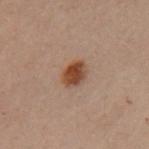Recorded during total-body skin imaging; not selected for excision or biopsy.
Longest diameter approximately 3 mm.
Captured under cross-polarized illumination.
Automated image analysis of the tile measured a footprint of about 5.5 mm², an eccentricity of roughly 0.7, and a symmetry-axis asymmetry near 0.1. The analysis additionally found a lesion color around L≈35 a*≈18 b*≈26 in CIELAB, roughly 11 lightness units darker than nearby skin, and a normalized lesion–skin contrast near 10.5.
A lesion tile, about 15 mm wide, cut from a 3D total-body photograph.
A female subject aged around 30.
On the left upper arm.